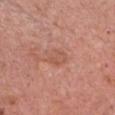| field | value |
|---|---|
| subject | female, about 60 years old |
| diameter | ≈2.5 mm |
| image-analysis metrics | an outline eccentricity of about 0.6 (0 = round, 1 = elongated) and two-axis asymmetry of about 0.25; a lesion color around L≈55 a*≈24 b*≈30 in CIELAB and a lesion–skin lightness drop of about 6; an automated nevus-likeness rating near 0 out of 100 and lesion-presence confidence of about 100/100 |
| tile lighting | white-light illumination |
| image | total-body-photography crop, ~15 mm field of view |
| location | the chest |